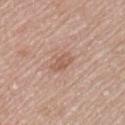Assessment: Imaged during a routine full-body skin examination; the lesion was not biopsied and no histopathology is available. Clinical summary: The lesion is on the left upper arm. A female patient aged 58 to 62. A roughly 15 mm field-of-view crop from a total-body skin photograph. The lesion's longest dimension is about 3 mm. Automated image analysis of the tile measured an eccentricity of roughly 0.85 and a symmetry-axis asymmetry near 0.25. It also reported a lesion color around L≈57 a*≈21 b*≈28 in CIELAB, a lesion–skin lightness drop of about 8, and a lesion-to-skin contrast of about 6 (normalized; higher = more distinct). It also reported border irregularity of about 3 on a 0–10 scale and a within-lesion color-variation index near 2.5/10. And it measured an automated nevus-likeness rating near 5 out of 100. The tile uses white-light illumination.